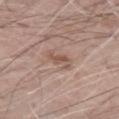{"biopsy_status": "not biopsied; imaged during a skin examination", "image": {"source": "total-body photography crop", "field_of_view_mm": 15}, "lesion_size": {"long_diameter_mm_approx": 3.0}, "patient": {"sex": "male", "age_approx": 70}, "site": "left forearm"}